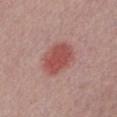workup=total-body-photography surveillance lesion; no biopsy | diameter=about 4.5 mm | automated metrics=a classifier nevus-likeness of about 100/100 | image source=15 mm crop, total-body photography | subject=male, about 30 years old.